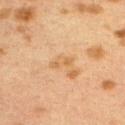A lesion tile, about 15 mm wide, cut from a 3D total-body photograph.
Captured under cross-polarized illumination.
A female patient roughly 40 years of age.
Located on the upper back.